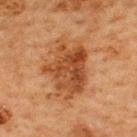Captured during whole-body skin photography for melanoma surveillance; the lesion was not biopsied. On the upper back. A male patient about 65 years old. A lesion tile, about 15 mm wide, cut from a 3D total-body photograph.